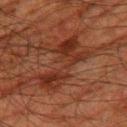The lesion was tiled from a total-body skin photograph and was not biopsied.
The patient is a male aged approximately 80.
Imaged with cross-polarized lighting.
On the right thigh.
A 15 mm crop from a total-body photograph taken for skin-cancer surveillance.